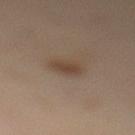Q: Is there a histopathology result?
A: imaged on a skin check; not biopsied
Q: Who is the patient?
A: female, aged around 40
Q: What is the anatomic site?
A: the left lower leg
Q: How was this image acquired?
A: total-body-photography crop, ~15 mm field of view